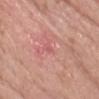Clinical summary:
The tile uses white-light illumination. The patient is a male aged 58–62. Automated image analysis of the tile measured a lesion color around L≈57 a*≈30 b*≈24 in CIELAB. The software also gave a border-irregularity rating of about 3/10, internal color variation of about 0 on a 0–10 scale, and peripheral color asymmetry of about 0. Longest diameter approximately 1.5 mm. Located on the chest. This image is a 15 mm lesion crop taken from a total-body photograph.